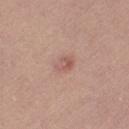Imaged during a routine full-body skin examination; the lesion was not biopsied and no histopathology is available.